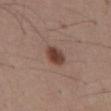<tbp_lesion>
  <biopsy_status>not biopsied; imaged during a skin examination</biopsy_status>
  <lesion_size>
    <long_diameter_mm_approx>3.0</long_diameter_mm_approx>
  </lesion_size>
  <patient>
    <sex>male</sex>
    <age_approx>55</age_approx>
  </patient>
  <image>
    <source>total-body photography crop</source>
    <field_of_view_mm>15</field_of_view_mm>
  </image>
  <automated_metrics>
    <border_irregularity_0_10>2.0</border_irregularity_0_10>
    <color_variation_0_10>4.0</color_variation_0_10>
    <peripheral_color_asymmetry>1.0</peripheral_color_asymmetry>
    <nevus_likeness_0_100>100</nevus_likeness_0_100>
    <lesion_detection_confidence_0_100>100</lesion_detection_confidence_0_100>
  </automated_metrics>
  <lighting>white-light</lighting>
  <site>abdomen</site>
</tbp_lesion>